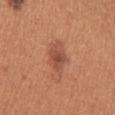No biopsy was performed on this lesion — it was imaged during a full skin examination and was not determined to be concerning. A female subject, approximately 40 years of age. The lesion is on the right upper arm. A close-up tile cropped from a whole-body skin photograph, about 15 mm across.